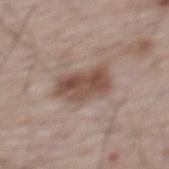No biopsy was performed on this lesion — it was imaged during a full skin examination and was not determined to be concerning.
The lesion is on the mid back.
The lesion's longest dimension is about 5 mm.
A 15 mm close-up extracted from a 3D total-body photography capture.
An algorithmic analysis of the crop reported a lesion area of about 14 mm², an outline eccentricity of about 0.75 (0 = round, 1 = elongated), and two-axis asymmetry of about 0.2. It also reported a lesion color around L≈50 a*≈16 b*≈25 in CIELAB and a lesion–skin lightness drop of about 11.
The patient is a male approximately 65 years of age.
Imaged with white-light lighting.